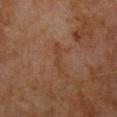biopsy status: imaged on a skin check; not biopsied
location: the chest
image-analysis metrics: an outline eccentricity of about 0.95 (0 = round, 1 = elongated); a lesion color around L≈31 a*≈17 b*≈25 in CIELAB, roughly 4 lightness units darker than nearby skin, and a normalized border contrast of about 5; border irregularity of about 6.5 on a 0–10 scale, a within-lesion color-variation index near 0/10, and peripheral color asymmetry of about 0
tile lighting: cross-polarized
patient: male, aged around 65
imaging modality: total-body-photography crop, ~15 mm field of view
size: ≈4 mm